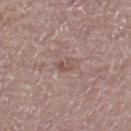This lesion was catalogued during total-body skin photography and was not selected for biopsy. A female subject aged around 65. Located on the left thigh. A 15 mm close-up tile from a total-body photography series done for melanoma screening.